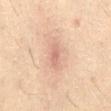Part of a total-body skin-imaging series; this lesion was reviewed on a skin check and was not flagged for biopsy.
The lesion-visualizer software estimated an average lesion color of about L≈59 a*≈20 b*≈26 (CIELAB), about 8 CIELAB-L* units darker than the surrounding skin, and a lesion-to-skin contrast of about 5.5 (normalized; higher = more distinct). The software also gave a border-irregularity rating of about 2.5/10 and a color-variation rating of about 1/10.
The lesion is located on the abdomen.
The tile uses cross-polarized illumination.
The patient is a male aged 48–52.
The recorded lesion diameter is about 2.5 mm.
Cropped from a whole-body photographic skin survey; the tile spans about 15 mm.